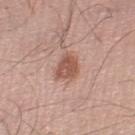follow-up=no biopsy performed (imaged during a skin exam).